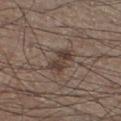workup = total-body-photography surveillance lesion; no biopsy
diameter = ~3.5 mm (longest diameter)
subject = male, aged around 35
acquisition = ~15 mm crop, total-body skin-cancer survey
anatomic site = the left lower leg